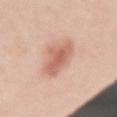{
  "biopsy_status": "not biopsied; imaged during a skin examination",
  "lighting": "white-light",
  "site": "right forearm",
  "automated_metrics": {
    "shape_asymmetry": 0.3,
    "cielab_L": 62,
    "cielab_a": 24,
    "cielab_b": 30,
    "vs_skin_darker_L": 12.0,
    "vs_skin_contrast_norm": 7.5
  },
  "image": {
    "source": "total-body photography crop",
    "field_of_view_mm": 15
  },
  "patient": {
    "sex": "female",
    "age_approx": 45
  }
}